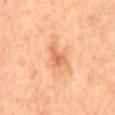notes=catalogued during a skin exam; not biopsied | tile lighting=cross-polarized | location=the mid back | subject=male, in their 70s | acquisition=15 mm crop, total-body photography | size=about 3.5 mm.